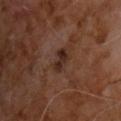  biopsy_status: not biopsied; imaged during a skin examination
  patient:
    sex: male
    age_approx: 60
  lighting: cross-polarized
  lesion_size:
    long_diameter_mm_approx: 3.0
  site: chest
  automated_metrics:
    area_mm2_approx: 4.0
    eccentricity: 0.75
    shape_asymmetry: 0.3
    cielab_L: 25
    cielab_a: 17
    cielab_b: 22
    vs_skin_darker_L: 9.0
  image:
    source: total-body photography crop
    field_of_view_mm: 15